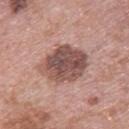<case>
  <biopsy_status>not biopsied; imaged during a skin examination</biopsy_status>
  <patient>
    <sex>female</sex>
    <age_approx>60</age_approx>
  </patient>
  <lesion_size>
    <long_diameter_mm_approx>5.5</long_diameter_mm_approx>
  </lesion_size>
  <site>upper back</site>
  <image>
    <source>total-body photography crop</source>
    <field_of_view_mm>15</field_of_view_mm>
  </image>
</case>